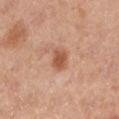Captured during whole-body skin photography for melanoma surveillance; the lesion was not biopsied. The lesion is on the left lower leg. A female patient, aged around 40. A lesion tile, about 15 mm wide, cut from a 3D total-body photograph.